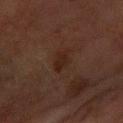<record>
<biopsy_status>not biopsied; imaged during a skin examination</biopsy_status>
<automated_metrics>
  <area_mm2_approx>4.0</area_mm2_approx>
  <eccentricity>0.7</eccentricity>
  <shape_asymmetry>0.3</shape_asymmetry>
  <cielab_L>19</cielab_L>
  <cielab_a>15</cielab_a>
  <cielab_b>20</cielab_b>
  <vs_skin_contrast_norm>7.0</vs_skin_contrast_norm>
</automated_metrics>
<patient>
  <sex>female</sex>
  <age_approx>70</age_approx>
</patient>
<lesion_size>
  <long_diameter_mm_approx>2.5</long_diameter_mm_approx>
</lesion_size>
<image>
  <source>total-body photography crop</source>
  <field_of_view_mm>15</field_of_view_mm>
</image>
<lighting>cross-polarized</lighting>
<site>right forearm</site>
</record>